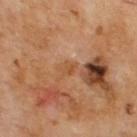Impression: The lesion was tiled from a total-body skin photograph and was not biopsied. Clinical summary: Automated image analysis of the tile measured a lesion color around L≈50 a*≈22 b*≈37 in CIELAB and a lesion-to-skin contrast of about 6 (normalized; higher = more distinct). The software also gave a border-irregularity rating of about 3/10 and a color-variation rating of about 3/10. The lesion's longest dimension is about 2.5 mm. A male patient, aged 68–72. A lesion tile, about 15 mm wide, cut from a 3D total-body photograph. On the upper back.